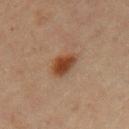{"biopsy_status": "not biopsied; imaged during a skin examination", "lighting": "cross-polarized", "patient": {"sex": "female", "age_approx": 45}, "image": {"source": "total-body photography crop", "field_of_view_mm": 15}, "automated_metrics": {"border_irregularity_0_10": 2.0, "color_variation_0_10": 4.0, "peripheral_color_asymmetry": 1.0}, "site": "arm", "lesion_size": {"long_diameter_mm_approx": 3.5}}